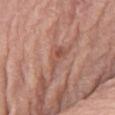{"biopsy_status": "not biopsied; imaged during a skin examination", "automated_metrics": {"vs_skin_darker_L": 8.0, "vs_skin_contrast_norm": 6.0, "border_irregularity_0_10": 6.5, "color_variation_0_10": 3.0, "peripheral_color_asymmetry": 1.0}, "lesion_size": {"long_diameter_mm_approx": 4.5}, "image": {"source": "total-body photography crop", "field_of_view_mm": 15}, "site": "chest", "patient": {"sex": "male", "age_approx": 80}, "lighting": "white-light"}